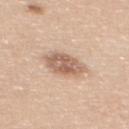Recorded during total-body skin imaging; not selected for excision or biopsy. Longest diameter approximately 4.5 mm. The subject is a female about 65 years old. From the mid back. A region of skin cropped from a whole-body photographic capture, roughly 15 mm wide. The total-body-photography lesion software estimated internal color variation of about 4.5 on a 0–10 scale and a peripheral color-asymmetry measure near 1.5.